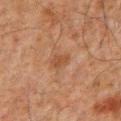Q: Was this lesion biopsied?
A: catalogued during a skin exam; not biopsied
Q: What are the patient's age and sex?
A: male, in their 60s
Q: What kind of image is this?
A: 15 mm crop, total-body photography
Q: What is the lesion's diameter?
A: about 2.5 mm
Q: What is the anatomic site?
A: the chest
Q: What lighting was used for the tile?
A: cross-polarized illumination